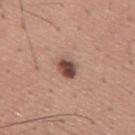This lesion was catalogued during total-body skin photography and was not selected for biopsy. The recorded lesion diameter is about 3 mm. The tile uses white-light illumination. The lesion is located on the mid back. Cropped from a total-body skin-imaging series; the visible field is about 15 mm. A male subject, roughly 45 years of age.